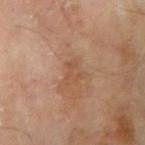The lesion was tiled from a total-body skin photograph and was not biopsied. A 15 mm close-up extracted from a 3D total-body photography capture. The lesion's longest dimension is about 3 mm. A male subject, aged around 65. From the right upper arm.